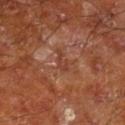Q: Was a biopsy performed?
A: no biopsy performed (imaged during a skin exam)